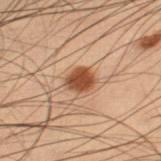subject=male, in their mid- to late 50s | lesion size=about 3 mm | anatomic site=the right thigh | image=15 mm crop, total-body photography.